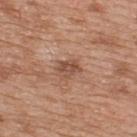follow-up — no biopsy performed (imaged during a skin exam)
acquisition — ~15 mm crop, total-body skin-cancer survey
lesion diameter — ≈3 mm
subject — male, in their 50s
anatomic site — the upper back
TBP lesion metrics — an outline eccentricity of about 0.7 (0 = round, 1 = elongated) and a symmetry-axis asymmetry near 0.2; an average lesion color of about L≈50 a*≈22 b*≈29 (CIELAB), a lesion–skin lightness drop of about 10, and a normalized lesion–skin contrast near 7; a border-irregularity index near 2.5/10, a color-variation rating of about 4/10, and peripheral color asymmetry of about 1.5; a nevus-likeness score of about 0/100